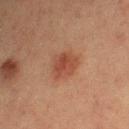Assessment: Recorded during total-body skin imaging; not selected for excision or biopsy. Clinical summary: A female patient, in their mid- to late 50s. Longest diameter approximately 3 mm. Located on the right upper arm. This is a cross-polarized tile. A region of skin cropped from a whole-body photographic capture, roughly 15 mm wide. Automated tile analysis of the lesion measured an automated nevus-likeness rating near 90 out of 100 and lesion-presence confidence of about 100/100.